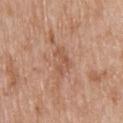biopsy status: imaged on a skin check; not biopsied
size: about 3.5 mm
tile lighting: white-light illumination
subject: male, in their 80s
site: the upper back
automated lesion analysis: a footprint of about 4 mm² and an outline eccentricity of about 0.85 (0 = round, 1 = elongated); a border-irregularity index near 7/10, internal color variation of about 0.5 on a 0–10 scale, and peripheral color asymmetry of about 0
image source: 15 mm crop, total-body photography